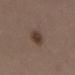follow-up: catalogued during a skin exam; not biopsied | lighting: white-light illumination | size: about 3 mm | patient: female, aged around 40 | site: the right lower leg | imaging modality: ~15 mm tile from a whole-body skin photo.